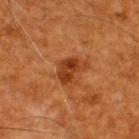Impression:
The lesion was photographed on a routine skin check and not biopsied; there is no pathology result.
Context:
On the upper back. A close-up tile cropped from a whole-body skin photograph, about 15 mm across. The lesion's longest dimension is about 3.5 mm. Automated tile analysis of the lesion measured a lesion area of about 6.5 mm², a shape eccentricity near 0.7, and a symmetry-axis asymmetry near 0.35. It also reported a normalized border contrast of about 8.5. The tile uses cross-polarized illumination. A male patient, about 60 years old.